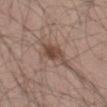| feature | finding |
|---|---|
| follow-up | catalogued during a skin exam; not biopsied |
| subject | male, approximately 45 years of age |
| image | total-body-photography crop, ~15 mm field of view |
| site | the left thigh |